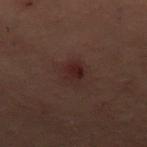notes = no biopsy performed (imaged during a skin exam)
automated lesion analysis = a border-irregularity index near 2.5/10, a color-variation rating of about 3.5/10, and radial color variation of about 1; a classifier nevus-likeness of about 40/100 and a lesion-detection confidence of about 100/100
body site = the left leg
image = 15 mm crop, total-body photography
lesion size = about 3 mm
subject = female, aged approximately 55